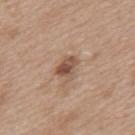Assessment:
Recorded during total-body skin imaging; not selected for excision or biopsy.
Background:
The patient is a female aged 38 to 42. A roughly 15 mm field-of-view crop from a total-body skin photograph. The lesion is on the upper back.